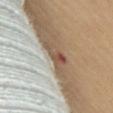{"site": "front of the torso", "automated_metrics": {"area_mm2_approx": 5.0, "eccentricity": 0.85, "shape_asymmetry": 0.6, "color_variation_0_10": 9.0, "peripheral_color_asymmetry": 3.5, "nevus_likeness_0_100": 0, "lesion_detection_confidence_0_100": 100}, "patient": {"sex": "female", "age_approx": 70}, "image": {"source": "total-body photography crop", "field_of_view_mm": 15}}